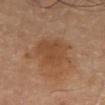Notes:
* follow-up · imaged on a skin check; not biopsied
* automated lesion analysis · about 6 CIELAB-L* units darker than the surrounding skin and a normalized border contrast of about 5.5; border irregularity of about 2.5 on a 0–10 scale, internal color variation of about 2.5 on a 0–10 scale, and a peripheral color-asymmetry measure near 1
* tile lighting · cross-polarized illumination
* image · total-body-photography crop, ~15 mm field of view
* patient · male, about 60 years old